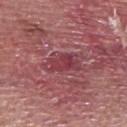Imaged during a routine full-body skin examination; the lesion was not biopsied and no histopathology is available. Cropped from a total-body skin-imaging series; the visible field is about 15 mm. Captured under white-light illumination. The subject is a male aged around 40. About 4.5 mm across. Located on the upper back.